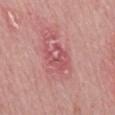Approximately 3.5 mm at its widest. Captured under white-light illumination. The lesion is located on the mid back. A close-up tile cropped from a whole-body skin photograph, about 15 mm across. The subject is a male aged 48–52.